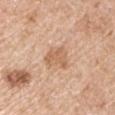Impression: No biopsy was performed on this lesion — it was imaged during a full skin examination and was not determined to be concerning. Context: Cropped from a total-body skin-imaging series; the visible field is about 15 mm. The lesion is located on the right upper arm. The tile uses white-light illumination. The subject is a male aged 68 to 72.